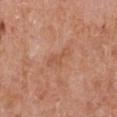Part of a total-body skin-imaging series; this lesion was reviewed on a skin check and was not flagged for biopsy.
A lesion tile, about 15 mm wide, cut from a 3D total-body photograph.
About 3.5 mm across.
The patient is a male about 65 years old.
From the arm.
This is a white-light tile.